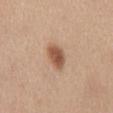<case>
  <patient>
    <sex>female</sex>
    <age_approx>40</age_approx>
  </patient>
  <image>
    <source>total-body photography crop</source>
    <field_of_view_mm>15</field_of_view_mm>
  </image>
  <site>mid back</site>
  <lighting>white-light</lighting>
  <lesion_size>
    <long_diameter_mm_approx>4.0</long_diameter_mm_approx>
  </lesion_size>
</case>